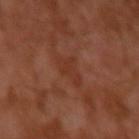From the left upper arm.
Automated tile analysis of the lesion measured an area of roughly 6 mm², an eccentricity of roughly 0.9, and a symmetry-axis asymmetry near 0.55. And it measured a border-irregularity index near 5.5/10, a within-lesion color-variation index near 1/10, and peripheral color asymmetry of about 0.5. It also reported a classifier nevus-likeness of about 0/100 and a lesion-detection confidence of about 95/100.
The patient is a male aged around 30.
A 15 mm close-up tile from a total-body photography series done for melanoma screening.